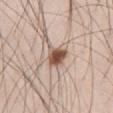Recorded during total-body skin imaging; not selected for excision or biopsy.
The tile uses white-light illumination.
An algorithmic analysis of the crop reported a lesion area of about 6 mm², a shape eccentricity near 0.65, and a symmetry-axis asymmetry near 0.25. The analysis additionally found a lesion–skin lightness drop of about 17 and a normalized lesion–skin contrast near 11. And it measured a detector confidence of about 100 out of 100 that the crop contains a lesion.
Measured at roughly 3 mm in maximum diameter.
A male patient, aged 33–37.
A 15 mm close-up extracted from a 3D total-body photography capture.
The lesion is on the right thigh.